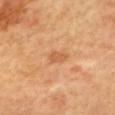Case summary:
* follow-up — imaged on a skin check; not biopsied
* tile lighting — cross-polarized
* subject — female, roughly 60 years of age
* imaging modality — 15 mm crop, total-body photography
* image-analysis metrics — an outline eccentricity of about 0.85 (0 = round, 1 = elongated) and a symmetry-axis asymmetry near 0.25; an average lesion color of about L≈61 a*≈26 b*≈43 (CIELAB) and a lesion–skin lightness drop of about 8; a border-irregularity index near 2.5/10, a within-lesion color-variation index near 1.5/10, and peripheral color asymmetry of about 0.5
* location — the upper back